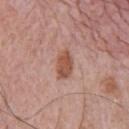The lesion was photographed on a routine skin check and not biopsied; there is no pathology result. From the chest. A male subject aged around 80. The total-body-photography lesion software estimated an average lesion color of about L≈53 a*≈23 b*≈29 (CIELAB), about 11 CIELAB-L* units darker than the surrounding skin, and a normalized border contrast of about 8. The software also gave a border-irregularity rating of about 2.5/10 and a within-lesion color-variation index near 3.5/10. Captured under white-light illumination. A roughly 15 mm field-of-view crop from a total-body skin photograph. The lesion's longest dimension is about 3.5 mm.